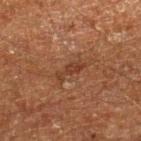Case summary:
– automated lesion analysis: an area of roughly 3.5 mm², an eccentricity of roughly 0.95, and a shape-asymmetry score of about 0.45 (0 = symmetric); a lesion color around L≈29 a*≈18 b*≈25 in CIELAB, a lesion–skin lightness drop of about 6, and a lesion-to-skin contrast of about 7 (normalized; higher = more distinct)
– image: ~15 mm tile from a whole-body skin photo
– location: the right lower leg
– patient: male, about 60 years old
– diameter: ≈3.5 mm
– illumination: cross-polarized illumination